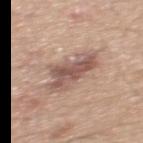The lesion is located on the back. The subject is a male roughly 45 years of age. A lesion tile, about 15 mm wide, cut from a 3D total-body photograph.